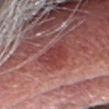biopsy_status: not biopsied; imaged during a skin examination
patient:
  sex: male
  age_approx: 75
lesion_size:
  long_diameter_mm_approx: 4.0
site: head or neck
image:
  source: total-body photography crop
  field_of_view_mm: 15
lighting: white-light
automated_metrics:
  eccentricity: 0.7
  shape_asymmetry: 0.35
  border_irregularity_0_10: 4.0
  peripheral_color_asymmetry: 1.5
  nevus_likeness_0_100: 0
  lesion_detection_confidence_0_100: 95